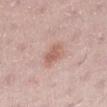image-analysis metrics: border irregularity of about 2.5 on a 0–10 scale, a within-lesion color-variation index near 3/10, and peripheral color asymmetry of about 1; a lesion-detection confidence of about 100/100 | lesion diameter: ~3 mm (longest diameter) | anatomic site: the left lower leg | patient: female, in their 50s | image: ~15 mm tile from a whole-body skin photo | lighting: white-light illumination.